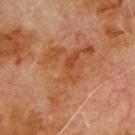TBP lesion metrics — a lesion color around L≈38 a*≈20 b*≈30 in CIELAB and roughly 5 lightness units darker than nearby skin; a border-irregularity index near 9.5/10 and peripheral color asymmetry of about 1.5; an automated nevus-likeness rating near 0 out of 100 | imaging modality — 15 mm crop, total-body photography | illumination — cross-polarized illumination | patient — male, approximately 80 years of age | body site — the front of the torso | lesion size — about 8.5 mm.